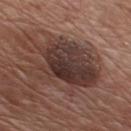- workup — catalogued during a skin exam; not biopsied
- size — ~8.5 mm (longest diameter)
- acquisition — total-body-photography crop, ~15 mm field of view
- lighting — white-light
- subject — male, in their mid-70s
- anatomic site — the chest